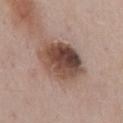Findings:
– workup: total-body-photography surveillance lesion; no biopsy
– patient: male, approximately 55 years of age
– tile lighting: white-light illumination
– site: the mid back
– TBP lesion metrics: a border-irregularity index near 2/10, internal color variation of about 10 on a 0–10 scale, and peripheral color asymmetry of about 3.5; a lesion-detection confidence of about 100/100
– image source: total-body-photography crop, ~15 mm field of view
– diameter: about 6.5 mm